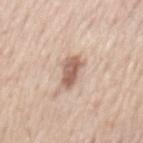Impression:
Imaged during a routine full-body skin examination; the lesion was not biopsied and no histopathology is available.
Clinical summary:
A 15 mm close-up extracted from a 3D total-body photography capture. Approximately 4 mm at its widest. The lesion-visualizer software estimated a mean CIELAB color near L≈60 a*≈19 b*≈27 and a normalized lesion–skin contrast near 9. The software also gave a classifier nevus-likeness of about 65/100 and lesion-presence confidence of about 100/100. The patient is a male aged 58–62. The lesion is on the mid back. Captured under white-light illumination.